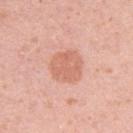The lesion was tiled from a total-body skin photograph and was not biopsied. The patient is a female roughly 30 years of age. Automated image analysis of the tile measured a footprint of about 11 mm², an eccentricity of roughly 0.15, and a shape-asymmetry score of about 0.15 (0 = symmetric). The analysis additionally found a border-irregularity index near 1.5/10, a within-lesion color-variation index near 2.5/10, and peripheral color asymmetry of about 1. The software also gave lesion-presence confidence of about 100/100. Captured under white-light illumination. About 4 mm across. The lesion is located on the left upper arm. This image is a 15 mm lesion crop taken from a total-body photograph.